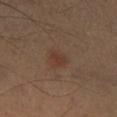<tbp_lesion>
  <image>
    <source>total-body photography crop</source>
    <field_of_view_mm>15</field_of_view_mm>
  </image>
  <site>left lower leg</site>
  <patient>
    <sex>male</sex>
    <age_approx>55</age_approx>
  </patient>
</tbp_lesion>